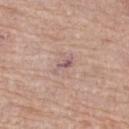On the leg. The total-body-photography lesion software estimated an eccentricity of roughly 0.9 and a symmetry-axis asymmetry near 0.5. The software also gave a mean CIELAB color near L≈57 a*≈19 b*≈19 and roughly 8 lightness units darker than nearby skin. And it measured an automated nevus-likeness rating near 0 out of 100. A roughly 15 mm field-of-view crop from a total-body skin photograph. Imaged with white-light lighting. A female subject aged around 70. Longest diameter approximately 3 mm.